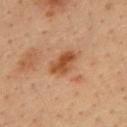<record>
<biopsy_status>not biopsied; imaged during a skin examination</biopsy_status>
<image>
  <source>total-body photography crop</source>
  <field_of_view_mm>15</field_of_view_mm>
</image>
<patient>
  <sex>male</sex>
  <age_approx>35</age_approx>
</patient>
<site>upper back</site>
</record>